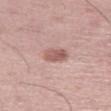lesion diameter: ~3 mm (longest diameter)
anatomic site: the leg
image: ~15 mm crop, total-body skin-cancer survey
patient: male, roughly 50 years of age
illumination: white-light